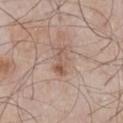workup: total-body-photography surveillance lesion; no biopsy | patient: male, about 55 years old | diameter: ≈4 mm | acquisition: total-body-photography crop, ~15 mm field of view | TBP lesion metrics: a lesion area of about 5.5 mm², an eccentricity of roughly 0.9, and a shape-asymmetry score of about 0.35 (0 = symmetric); border irregularity of about 4.5 on a 0–10 scale, internal color variation of about 5.5 on a 0–10 scale, and peripheral color asymmetry of about 1.5; an automated nevus-likeness rating near 0 out of 100 and a lesion-detection confidence of about 100/100 | site: the chest.